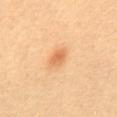biopsy status=imaged on a skin check; not biopsied | lesion diameter=about 2.5 mm | subject=female, in their mid-40s | image=~15 mm crop, total-body skin-cancer survey | anatomic site=the back.